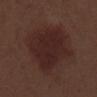<record>
<biopsy_status>not biopsied; imaged during a skin examination</biopsy_status>
<site>right lower leg</site>
<patient>
  <sex>male</sex>
  <age_approx>70</age_approx>
</patient>
<lesion_size>
  <long_diameter_mm_approx>7.0</long_diameter_mm_approx>
</lesion_size>
<image>
  <source>total-body photography crop</source>
  <field_of_view_mm>15</field_of_view_mm>
</image>
</record>